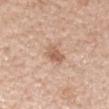Findings:
- follow-up · total-body-photography surveillance lesion; no biopsy
- body site · the right forearm
- automated metrics · about 10 CIELAB-L* units darker than the surrounding skin
- tile lighting · white-light
- lesion diameter · about 3 mm
- image source · ~15 mm tile from a whole-body skin photo
- subject · female, aged approximately 45